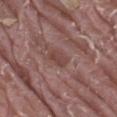Assessment: Imaged during a routine full-body skin examination; the lesion was not biopsied and no histopathology is available. Background: The tile uses white-light illumination. The lesion is on the right thigh. This image is a 15 mm lesion crop taken from a total-body photograph. About 3 mm across. A male patient in their 40s.